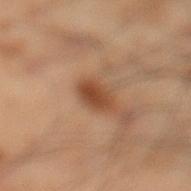workup = imaged on a skin check; not biopsied
subject = male, approximately 50 years of age
imaging modality = ~15 mm tile from a whole-body skin photo
body site = the left lower leg